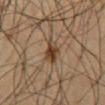Captured during whole-body skin photography for melanoma surveillance; the lesion was not biopsied.
The lesion is located on the abdomen.
A male subject aged around 60.
The tile uses cross-polarized illumination.
Cropped from a whole-body photographic skin survey; the tile spans about 15 mm.
About 4 mm across.
Automated image analysis of the tile measured a border-irregularity rating of about 3.5/10. And it measured a lesion-detection confidence of about 100/100.